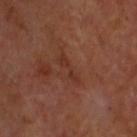Clinical summary:
From the head or neck. The total-body-photography lesion software estimated a lesion color around L≈31 a*≈22 b*≈26 in CIELAB and a normalized border contrast of about 5.5. A male subject, aged around 60. The tile uses cross-polarized illumination. A region of skin cropped from a whole-body photographic capture, roughly 15 mm wide.